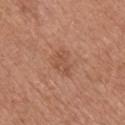Imaged during a routine full-body skin examination; the lesion was not biopsied and no histopathology is available.